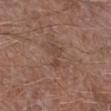patient: male, approximately 70 years of age | TBP lesion metrics: internal color variation of about 0.5 on a 0–10 scale and radial color variation of about 0; a nevus-likeness score of about 0/100 | size: ≈3 mm | acquisition: ~15 mm tile from a whole-body skin photo | location: the right lower leg | lighting: white-light.